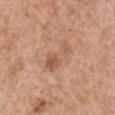Assessment:
No biopsy was performed on this lesion — it was imaged during a full skin examination and was not determined to be concerning.